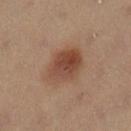Q: Was a biopsy performed?
A: total-body-photography surveillance lesion; no biopsy
Q: Lesion location?
A: the left lower leg
Q: Automated lesion metrics?
A: a footprint of about 13 mm², a shape eccentricity near 0.7, and a symmetry-axis asymmetry near 0.1; a nevus-likeness score of about 100/100 and a detector confidence of about 100 out of 100 that the crop contains a lesion
Q: What is the lesion's diameter?
A: about 5 mm
Q: How was the tile lit?
A: cross-polarized
Q: How was this image acquired?
A: 15 mm crop, total-body photography
Q: Patient demographics?
A: female, aged 33–37